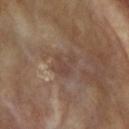Assessment:
This lesion was catalogued during total-body skin photography and was not selected for biopsy.
Context:
A female patient, in their mid-70s. The lesion-visualizer software estimated an area of roughly 6.5 mm² and an outline eccentricity of about 0.65 (0 = round, 1 = elongated). The software also gave a classifier nevus-likeness of about 0/100 and a detector confidence of about 90 out of 100 that the crop contains a lesion. The lesion is on the right upper arm. Cropped from a whole-body photographic skin survey; the tile spans about 15 mm.